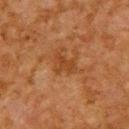notes: no biopsy performed (imaged during a skin exam) | site: the upper back | illumination: cross-polarized illumination | image source: 15 mm crop, total-body photography | patient: male, about 65 years old.